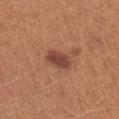<tbp_lesion>
  <biopsy_status>not biopsied; imaged during a skin examination</biopsy_status>
  <image>
    <source>total-body photography crop</source>
    <field_of_view_mm>15</field_of_view_mm>
  </image>
  <site>left forearm</site>
  <lesion_size>
    <long_diameter_mm_approx>3.5</long_diameter_mm_approx>
  </lesion_size>
  <automated_metrics>
    <area_mm2_approx>6.0</area_mm2_approx>
    <shape_asymmetry>0.2</shape_asymmetry>
  </automated_metrics>
  <patient>
    <sex>female</sex>
    <age_approx>25</age_approx>
  </patient>
</tbp_lesion>